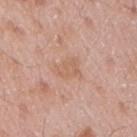biopsy status = no biopsy performed (imaged during a skin exam); tile lighting = white-light; location = the arm; image = ~15 mm tile from a whole-body skin photo; image-analysis metrics = a border-irregularity rating of about 3/10 and radial color variation of about 0.5; diameter = ≈3 mm; subject = male, aged 53–57.